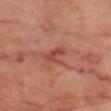The lesion was tiled from a total-body skin photograph and was not biopsied. A 15 mm close-up tile from a total-body photography series done for melanoma screening. The subject is a male roughly 70 years of age. The lesion is located on the left thigh.